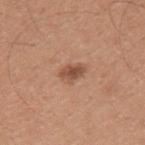{"biopsy_status": "not biopsied; imaged during a skin examination", "lighting": "white-light", "lesion_size": {"long_diameter_mm_approx": 3.0}, "image": {"source": "total-body photography crop", "field_of_view_mm": 15}, "site": "left upper arm", "patient": {"sex": "male", "age_approx": 30}}